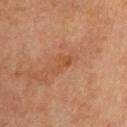  biopsy_status: not biopsied; imaged during a skin examination
  site: left upper arm
  patient:
    sex: male
    age_approx: 65
  lesion_size:
    long_diameter_mm_approx: 2.5
  image:
    source: total-body photography crop
    field_of_view_mm: 15
  lighting: cross-polarized